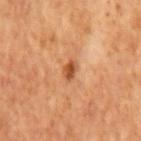follow-up — no biopsy performed (imaged during a skin exam)
patient — male, in their mid-60s
imaging modality — 15 mm crop, total-body photography
automated lesion analysis — a footprint of about 3 mm², a shape eccentricity near 0.8, and a shape-asymmetry score of about 0.25 (0 = symmetric); a border-irregularity rating of about 2.5/10, a within-lesion color-variation index near 2.5/10, and a peripheral color-asymmetry measure near 1